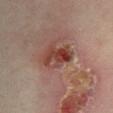Q: Was this lesion biopsied?
A: catalogued during a skin exam; not biopsied
Q: What kind of image is this?
A: 15 mm crop, total-body photography
Q: How large is the lesion?
A: about 4.5 mm
Q: What is the anatomic site?
A: the leg
Q: How was the tile lit?
A: cross-polarized illumination
Q: Automated lesion metrics?
A: a border-irregularity rating of about 3/10, internal color variation of about 9.5 on a 0–10 scale, and a peripheral color-asymmetry measure near 3.5; a nevus-likeness score of about 0/100 and lesion-presence confidence of about 100/100
Q: Patient demographics?
A: female, approximately 35 years of age